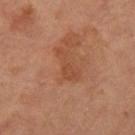The lesion was photographed on a routine skin check and not biopsied; there is no pathology result.
Approximately 4 mm at its widest.
From the right thigh.
A 15 mm close-up tile from a total-body photography series done for melanoma screening.
A female subject, aged around 65.